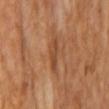lighting = cross-polarized | automated metrics = a lesion color around L≈45 a*≈23 b*≈34 in CIELAB; a nevus-likeness score of about 0/100 and lesion-presence confidence of about 100/100 | subject = male, approximately 65 years of age | imaging modality = total-body-photography crop, ~15 mm field of view | lesion size = about 3.5 mm.